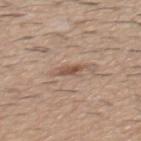Q: Is there a histopathology result?
A: imaged on a skin check; not biopsied
Q: Automated lesion metrics?
A: a footprint of about 2.5 mm², an eccentricity of roughly 0.85, and a symmetry-axis asymmetry near 0.3; a border-irregularity rating of about 3.5/10, internal color variation of about 0 on a 0–10 scale, and radial color variation of about 0; a lesion-detection confidence of about 100/100
Q: Patient demographics?
A: male, about 60 years old
Q: What is the lesion's diameter?
A: ≈2.5 mm
Q: Lesion location?
A: the mid back
Q: What lighting was used for the tile?
A: white-light
Q: What kind of image is this?
A: ~15 mm crop, total-body skin-cancer survey